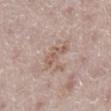Q: Is there a histopathology result?
A: total-body-photography surveillance lesion; no biopsy
Q: How was this image acquired?
A: total-body-photography crop, ~15 mm field of view
Q: What is the anatomic site?
A: the right lower leg
Q: What are the patient's age and sex?
A: female, aged 48–52
Q: What lighting was used for the tile?
A: white-light illumination